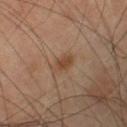The lesion was photographed on a routine skin check and not biopsied; there is no pathology result.
Imaged with cross-polarized lighting.
Located on the leg.
The lesion-visualizer software estimated an eccentricity of roughly 0.85 and a shape-asymmetry score of about 0.3 (0 = symmetric). It also reported a mean CIELAB color near L≈44 a*≈18 b*≈30, a lesion–skin lightness drop of about 8, and a normalized border contrast of about 7. The software also gave a border-irregularity rating of about 3/10, internal color variation of about 1.5 on a 0–10 scale, and a peripheral color-asymmetry measure near 0.5.
A male subject aged approximately 70.
A 15 mm close-up extracted from a 3D total-body photography capture.